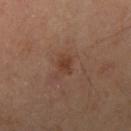notes: catalogued during a skin exam; not biopsied | anatomic site: the right upper arm | patient: male, approximately 55 years of age | image source: ~15 mm tile from a whole-body skin photo.